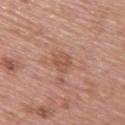biopsy status: no biopsy performed (imaged during a skin exam)
diameter: ≈2.5 mm
site: the upper back
acquisition: ~15 mm crop, total-body skin-cancer survey
subject: female, roughly 60 years of age
illumination: white-light